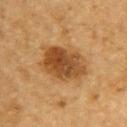<case>
<biopsy_status>not biopsied; imaged during a skin examination</biopsy_status>
<site>upper back</site>
<patient>
  <sex>male</sex>
  <age_approx>85+</age_approx>
</patient>
<image>
  <source>total-body photography crop</source>
  <field_of_view_mm>15</field_of_view_mm>
</image>
<lesion_size>
  <long_diameter_mm_approx>5.5</long_diameter_mm_approx>
</lesion_size>
</case>